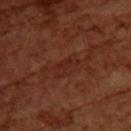Q: How was the tile lit?
A: cross-polarized
Q: Lesion size?
A: about 3 mm
Q: Patient demographics?
A: female, aged approximately 55
Q: What is the anatomic site?
A: the upper back
Q: What is the imaging modality?
A: total-body-photography crop, ~15 mm field of view